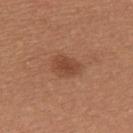follow-up = imaged on a skin check; not biopsied | patient = female, roughly 25 years of age | image = total-body-photography crop, ~15 mm field of view | automated metrics = a lesion color around L≈45 a*≈23 b*≈32 in CIELAB, a lesion–skin lightness drop of about 9, and a normalized border contrast of about 7 | anatomic site = the upper back.